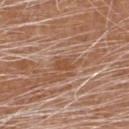workup=no biopsy performed (imaged during a skin exam)
lesion diameter=≈3 mm
acquisition=~15 mm crop, total-body skin-cancer survey
patient=male, in their mid- to late 50s
body site=the head or neck
tile lighting=white-light illumination